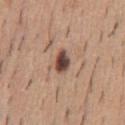biopsy status — no biopsy performed (imaged during a skin exam) | body site — the chest | tile lighting — white-light | image — total-body-photography crop, ~15 mm field of view | subject — male, aged 38 to 42 | size — about 3 mm.